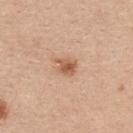Q: Is there a histopathology result?
A: no biopsy performed (imaged during a skin exam)
Q: Lesion location?
A: the upper back
Q: How large is the lesion?
A: about 2.5 mm
Q: What is the imaging modality?
A: ~15 mm tile from a whole-body skin photo
Q: What did automated image analysis measure?
A: a footprint of about 4.5 mm², an outline eccentricity of about 0.6 (0 = round, 1 = elongated), and two-axis asymmetry of about 0.25; a lesion color around L≈58 a*≈22 b*≈33 in CIELAB and roughly 11 lightness units darker than nearby skin
Q: What are the patient's age and sex?
A: female, approximately 40 years of age